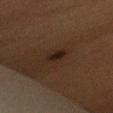Clinical impression: The lesion was photographed on a routine skin check and not biopsied; there is no pathology result. Context: Measured at roughly 3 mm in maximum diameter. A female subject, aged around 55. A 15 mm crop from a total-body photograph taken for skin-cancer surveillance. From the left upper arm.